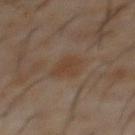biopsy status: catalogued during a skin exam; not biopsied
site: the abdomen
patient: male, aged 58–62
acquisition: total-body-photography crop, ~15 mm field of view